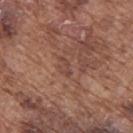workup: catalogued during a skin exam; not biopsied
patient: male, in their mid-70s
acquisition: total-body-photography crop, ~15 mm field of view
lighting: white-light
lesion size: ≈2.5 mm
body site: the upper back